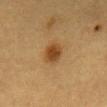Impression: This lesion was catalogued during total-body skin photography and was not selected for biopsy. Clinical summary: Located on the back. This image is a 15 mm lesion crop taken from a total-body photograph. A female patient aged approximately 55. This is a cross-polarized tile. Approximately 3 mm at its widest.